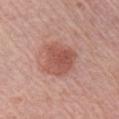{
  "biopsy_status": "not biopsied; imaged during a skin examination",
  "patient": {
    "sex": "male",
    "age_approx": 55
  },
  "site": "right upper arm",
  "image": {
    "source": "total-body photography crop",
    "field_of_view_mm": 15
  }
}